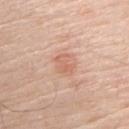Clinical impression:
Recorded during total-body skin imaging; not selected for excision or biopsy.
Context:
The lesion's longest dimension is about 2.5 mm. This image is a 15 mm lesion crop taken from a total-body photograph. An algorithmic analysis of the crop reported an area of roughly 4 mm², a shape eccentricity near 0.7, and two-axis asymmetry of about 0.45. The software also gave an automated nevus-likeness rating near 15 out of 100. A male subject aged approximately 70. On the right upper arm. This is a white-light tile.